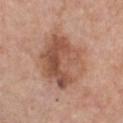Impression:
The lesion was photographed on a routine skin check and not biopsied; there is no pathology result.
Clinical summary:
A 15 mm crop from a total-body photograph taken for skin-cancer surveillance. Measured at roughly 7 mm in maximum diameter. The patient is a male aged 58 to 62. The lesion is located on the chest. The tile uses white-light illumination.